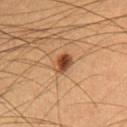The lesion was photographed on a routine skin check and not biopsied; there is no pathology result.
The tile uses cross-polarized illumination.
An algorithmic analysis of the crop reported an eccentricity of roughly 0.65 and two-axis asymmetry of about 0.2. The analysis additionally found a border-irregularity rating of about 1.5/10, a within-lesion color-variation index near 4.5/10, and peripheral color asymmetry of about 1.5.
A male patient, approximately 55 years of age.
Located on the upper back.
A 15 mm close-up tile from a total-body photography series done for melanoma screening.
Measured at roughly 2.5 mm in maximum diameter.